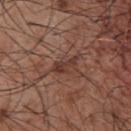Findings:
* follow-up: catalogued during a skin exam; not biopsied
* body site: the chest
* illumination: white-light illumination
* acquisition: ~15 mm tile from a whole-body skin photo
* patient: male, aged around 55